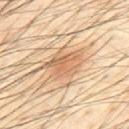{
  "image": {
    "source": "total-body photography crop",
    "field_of_view_mm": 15
  },
  "site": "upper back",
  "patient": {
    "sex": "male",
    "age_approx": 40
  }
}